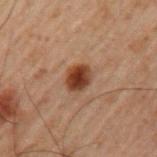Context:
The lesion is on the left upper arm. Cropped from a total-body skin-imaging series; the visible field is about 15 mm. A male patient in their 60s. This is a cross-polarized tile.